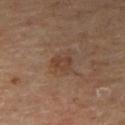Captured during whole-body skin photography for melanoma surveillance; the lesion was not biopsied. A lesion tile, about 15 mm wide, cut from a 3D total-body photograph. The lesion is located on the right thigh. The tile uses cross-polarized illumination. A male subject, roughly 65 years of age.